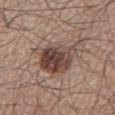The lesion was photographed on a routine skin check and not biopsied; there is no pathology result.
Located on the left lower leg.
A close-up tile cropped from a whole-body skin photograph, about 15 mm across.
The subject is a male aged 58–62.